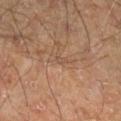* follow-up: imaged on a skin check; not biopsied
* location: the right lower leg
* patient: male, aged around 60
* tile lighting: cross-polarized illumination
* acquisition: ~15 mm crop, total-body skin-cancer survey
* TBP lesion metrics: an area of roughly 1 mm², a shape eccentricity near 0.55, and a symmetry-axis asymmetry near 0.25; an average lesion color of about L≈45 a*≈16 b*≈26 (CIELAB) and a normalized lesion–skin contrast near 4.5; border irregularity of about 2 on a 0–10 scale, a within-lesion color-variation index near 0/10, and a peripheral color-asymmetry measure near 0